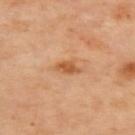Assessment: This lesion was catalogued during total-body skin photography and was not selected for biopsy. Background: Imaged with cross-polarized lighting. On the upper back. A 15 mm crop from a total-body photograph taken for skin-cancer surveillance. Measured at roughly 3 mm in maximum diameter.